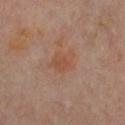{"biopsy_status": "not biopsied; imaged during a skin examination", "site": "front of the torso", "image": {"source": "total-body photography crop", "field_of_view_mm": 15}, "patient": {"sex": "female", "age_approx": 60}}